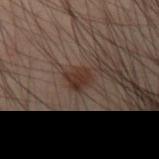Assessment:
The lesion was tiled from a total-body skin photograph and was not biopsied.
Acquisition and patient details:
Cropped from a whole-body photographic skin survey; the tile spans about 15 mm. On the right forearm. Approximately 2.5 mm at its widest. The subject is a male aged 38 to 42. This is a cross-polarized tile.